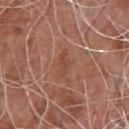  biopsy_status: not biopsied; imaged during a skin examination
  image:
    source: total-body photography crop
    field_of_view_mm: 15
  lighting: white-light
  patient:
    sex: male
    age_approx: 75
  site: chest